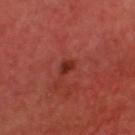Recorded during total-body skin imaging; not selected for excision or biopsy. A close-up tile cropped from a whole-body skin photograph, about 15 mm across. A male patient aged approximately 60. Imaged with cross-polarized lighting. The lesion is on the head or neck.